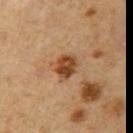Imaged during a routine full-body skin examination; the lesion was not biopsied and no histopathology is available.